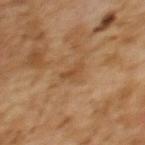• follow-up: no biopsy performed (imaged during a skin exam)
• subject: female, aged 58 to 62
• tile lighting: cross-polarized illumination
• size: about 3 mm
• imaging modality: total-body-photography crop, ~15 mm field of view
• site: the upper back